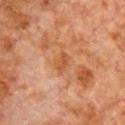Q: Is there a histopathology result?
A: imaged on a skin check; not biopsied
Q: What is the anatomic site?
A: the front of the torso
Q: Automated lesion metrics?
A: a border-irregularity rating of about 2.5/10 and internal color variation of about 1.5 on a 0–10 scale; a nevus-likeness score of about 0/100 and a lesion-detection confidence of about 100/100
Q: Patient demographics?
A: male, in their 80s
Q: What kind of image is this?
A: ~15 mm tile from a whole-body skin photo
Q: How large is the lesion?
A: about 2.5 mm
Q: Illumination type?
A: cross-polarized illumination